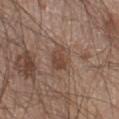Background:
The recorded lesion diameter is about 3 mm. The lesion is on the left lower leg. Automated tile analysis of the lesion measured a shape-asymmetry score of about 0.25 (0 = symmetric). The analysis additionally found a lesion–skin lightness drop of about 8 and a normalized lesion–skin contrast near 7. The analysis additionally found border irregularity of about 3 on a 0–10 scale, a within-lesion color-variation index near 2.5/10, and peripheral color asymmetry of about 1. A male subject aged approximately 20. The tile uses white-light illumination. A 15 mm close-up tile from a total-body photography series done for melanoma screening.